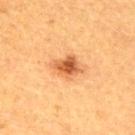This lesion was catalogued during total-body skin photography and was not selected for biopsy. From the upper back. Automated image analysis of the tile measured an area of roughly 7.5 mm² and two-axis asymmetry of about 0.25. It also reported about 12 CIELAB-L* units darker than the surrounding skin and a normalized border contrast of about 8.5. The recorded lesion diameter is about 4 mm. The subject is a male aged around 50. Imaged with cross-polarized lighting. Cropped from a total-body skin-imaging series; the visible field is about 15 mm.